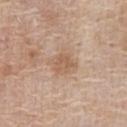Clinical impression: This lesion was catalogued during total-body skin photography and was not selected for biopsy. Image and clinical context: Imaged with white-light lighting. Automated tile analysis of the lesion measured an eccentricity of roughly 0.5 and two-axis asymmetry of about 0.15. The analysis additionally found a lesion color around L≈58 a*≈18 b*≈31 in CIELAB. From the chest. Measured at roughly 3 mm in maximum diameter. A close-up tile cropped from a whole-body skin photograph, about 15 mm across. A female subject, aged 58 to 62.